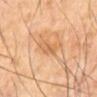The subject is a male in their mid- to late 60s.
A 15 mm close-up extracted from a 3D total-body photography capture.
Located on the front of the torso.